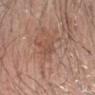* follow-up — imaged on a skin check; not biopsied
* diameter — ≈3.5 mm
* location — the right upper arm
* image source — total-body-photography crop, ~15 mm field of view
* tile lighting — white-light illumination
* automated metrics — a footprint of about 4.5 mm², an eccentricity of roughly 0.85, and a shape-asymmetry score of about 0.4 (0 = symmetric); roughly 6 lightness units darker than nearby skin and a lesion-to-skin contrast of about 5 (normalized; higher = more distinct); an automated nevus-likeness rating near 0 out of 100 and lesion-presence confidence of about 95/100
* patient — male, aged around 70